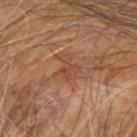Q: Was this lesion biopsied?
A: total-body-photography surveillance lesion; no biopsy
Q: How was the tile lit?
A: cross-polarized
Q: What is the lesion's diameter?
A: about 4 mm
Q: What is the anatomic site?
A: the left arm
Q: What are the patient's age and sex?
A: male, approximately 60 years of age
Q: What kind of image is this?
A: ~15 mm crop, total-body skin-cancer survey